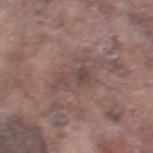The lesion was photographed on a routine skin check and not biopsied; there is no pathology result. The lesion-visualizer software estimated a footprint of about 8.5 mm², an eccentricity of roughly 0.85, and a shape-asymmetry score of about 0.55 (0 = symmetric). And it measured border irregularity of about 7.5 on a 0–10 scale, internal color variation of about 3.5 on a 0–10 scale, and a peripheral color-asymmetry measure near 1. Longest diameter approximately 5 mm. Located on the right thigh. A male subject roughly 75 years of age. The tile uses white-light illumination. A close-up tile cropped from a whole-body skin photograph, about 15 mm across.